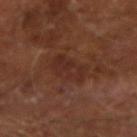follow-up: catalogued during a skin exam; not biopsied | tile lighting: cross-polarized illumination | anatomic site: the right forearm | subject: male, about 65 years old | automated metrics: a footprint of about 8 mm², an outline eccentricity of about 0.9 (0 = round, 1 = elongated), and a shape-asymmetry score of about 0.3 (0 = symmetric); a lesion color around L≈27 a*≈20 b*≈23 in CIELAB, a lesion–skin lightness drop of about 5, and a lesion-to-skin contrast of about 6 (normalized; higher = more distinct); a border-irregularity index near 4/10, internal color variation of about 2.5 on a 0–10 scale, and a peripheral color-asymmetry measure near 1 | acquisition: ~15 mm crop, total-body skin-cancer survey | lesion diameter: ~4.5 mm (longest diameter).